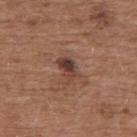Background:
The lesion's longest dimension is about 3.5 mm. A male patient, aged around 75. From the upper back. An algorithmic analysis of the crop reported a footprint of about 5 mm² and an outline eccentricity of about 0.8 (0 = round, 1 = elongated). The software also gave a border-irregularity rating of about 3/10 and a peripheral color-asymmetry measure near 2.5. The analysis additionally found a classifier nevus-likeness of about 15/100 and lesion-presence confidence of about 100/100. The tile uses white-light illumination. This image is a 15 mm lesion crop taken from a total-body photograph.